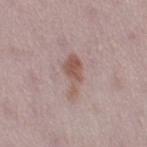notes=no biopsy performed (imaged during a skin exam) | patient=female, in their 30s | tile lighting=white-light | location=the right thigh | image=~15 mm tile from a whole-body skin photo | image-analysis metrics=a footprint of about 7 mm², a shape eccentricity near 0.9, and a symmetry-axis asymmetry near 0.45; an average lesion color of about L≈54 a*≈18 b*≈23 (CIELAB) and a normalized border contrast of about 7.5; a border-irregularity index near 5.5/10, internal color variation of about 2.5 on a 0–10 scale, and radial color variation of about 1.